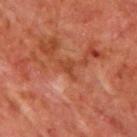Part of a total-body skin-imaging series; this lesion was reviewed on a skin check and was not flagged for biopsy. A male patient in their mid-60s. On the upper back. A 15 mm close-up tile from a total-body photography series done for melanoma screening.